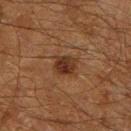biopsy status: imaged on a skin check; not biopsied | diameter: ≈3 mm | location: the right lower leg | subject: male, aged around 60 | automated metrics: about 8 CIELAB-L* units darker than the surrounding skin and a lesion-to-skin contrast of about 9 (normalized; higher = more distinct); a border-irregularity index near 2/10, internal color variation of about 4 on a 0–10 scale, and a peripheral color-asymmetry measure near 1 | image source: 15 mm crop, total-body photography.